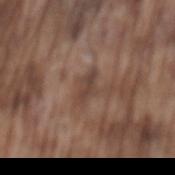– notes: catalogued during a skin exam; not biopsied
– acquisition: total-body-photography crop, ~15 mm field of view
– lesion size: ≈3 mm
– site: the mid back
– subject: male, about 75 years old
– lighting: white-light illumination